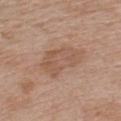No biopsy was performed on this lesion — it was imaged during a full skin examination and was not determined to be concerning.
A region of skin cropped from a whole-body photographic capture, roughly 15 mm wide.
The lesion is located on the chest.
The tile uses white-light illumination.
A male patient, approximately 65 years of age.
Measured at roughly 5.5 mm in maximum diameter.